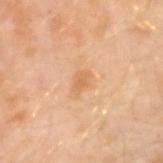Notes:
* notes · catalogued during a skin exam; not biopsied
* imaging modality · ~15 mm crop, total-body skin-cancer survey
* image-analysis metrics · a lesion area of about 3.5 mm² and an outline eccentricity of about 0.75 (0 = round, 1 = elongated); a mean CIELAB color near L≈64 a*≈21 b*≈39; a border-irregularity rating of about 2/10, a within-lesion color-variation index near 2/10, and peripheral color asymmetry of about 0.5; an automated nevus-likeness rating near 5 out of 100
* site · the left arm
* subject · male, aged around 30